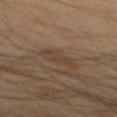Captured during whole-body skin photography for melanoma surveillance; the lesion was not biopsied. Captured under cross-polarized illumination. On the mid back. A male patient, about 45 years old. Automated image analysis of the tile measured a footprint of about 3.5 mm², a shape eccentricity near 0.85, and two-axis asymmetry of about 0.5. And it measured a mean CIELAB color near L≈31 a*≈12 b*≈22 and roughly 4 lightness units darker than nearby skin. It also reported an automated nevus-likeness rating near 0 out of 100 and lesion-presence confidence of about 100/100. A close-up tile cropped from a whole-body skin photograph, about 15 mm across. About 3 mm across.